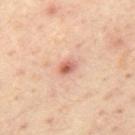Findings:
- biopsy status — no biopsy performed (imaged during a skin exam)
- patient — male, about 45 years old
- image source — total-body-photography crop, ~15 mm field of view
- lesion size — ≈2 mm
- anatomic site — the mid back
- illumination — cross-polarized illumination